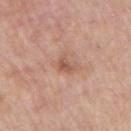{
  "biopsy_status": "not biopsied; imaged during a skin examination",
  "lesion_size": {
    "long_diameter_mm_approx": 2.5
  },
  "image": {
    "source": "total-body photography crop",
    "field_of_view_mm": 15
  },
  "site": "right upper arm",
  "lighting": "white-light",
  "patient": {
    "sex": "male",
    "age_approx": 75
  }
}